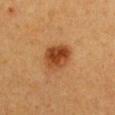biopsy status: imaged on a skin check; not biopsied.